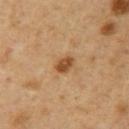| key | value |
|---|---|
| workup | imaged on a skin check; not biopsied |
| body site | the right upper arm |
| image-analysis metrics | an average lesion color of about L≈48 a*≈21 b*≈37 (CIELAB), about 12 CIELAB-L* units darker than the surrounding skin, and a normalized lesion–skin contrast near 9; an automated nevus-likeness rating near 90 out of 100 |
| patient | male, aged 53 to 57 |
| lesion diameter | about 2.5 mm |
| acquisition | ~15 mm tile from a whole-body skin photo |